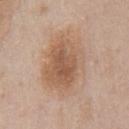<case>
<biopsy_status>not biopsied; imaged during a skin examination</biopsy_status>
<lesion_size>
  <long_diameter_mm_approx>8.5</long_diameter_mm_approx>
</lesion_size>
<site>front of the torso</site>
<image>
  <source>total-body photography crop</source>
  <field_of_view_mm>15</field_of_view_mm>
</image>
<automated_metrics>
  <eccentricity>0.75</eccentricity>
  <shape_asymmetry>0.2</shape_asymmetry>
</automated_metrics>
<patient>
  <sex>male</sex>
  <age_approx>60</age_approx>
</patient>
</case>